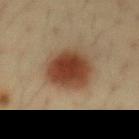Notes:
– patient — male, about 35 years old
– imaging modality — total-body-photography crop, ~15 mm field of view
– diameter — ≈5.5 mm
– site — the abdomen
– illumination — cross-polarized